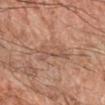Notes:
* notes · no biopsy performed (imaged during a skin exam)
* lighting · cross-polarized illumination
* imaging modality · 15 mm crop, total-body photography
* size · ≈4.5 mm
* image-analysis metrics · an average lesion color of about L≈50 a*≈19 b*≈28 (CIELAB), roughly 7 lightness units darker than nearby skin, and a lesion-to-skin contrast of about 5 (normalized; higher = more distinct); a border-irregularity rating of about 4.5/10, a within-lesion color-variation index near 3/10, and a peripheral color-asymmetry measure near 1; a nevus-likeness score of about 0/100 and a detector confidence of about 70 out of 100 that the crop contains a lesion
* anatomic site · the right forearm
* patient · male, about 60 years old